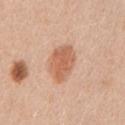Q: Was this lesion biopsied?
A: no biopsy performed (imaged during a skin exam)
Q: What is the anatomic site?
A: the left upper arm
Q: Patient demographics?
A: female, approximately 45 years of age
Q: What is the imaging modality?
A: ~15 mm crop, total-body skin-cancer survey